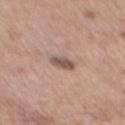biopsy_status: not biopsied; imaged during a skin examination
site: back
image:
  source: total-body photography crop
  field_of_view_mm: 15
patient:
  sex: male
  age_approx: 55